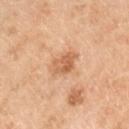{"biopsy_status": "not biopsied; imaged during a skin examination", "site": "right lower leg", "lesion_size": {"long_diameter_mm_approx": 3.5}, "automated_metrics": {"eccentricity": 0.75, "shape_asymmetry": 0.3, "color_variation_0_10": 4.5, "peripheral_color_asymmetry": 1.5, "nevus_likeness_0_100": 0, "lesion_detection_confidence_0_100": 100}, "lighting": "cross-polarized", "image": {"source": "total-body photography crop", "field_of_view_mm": 15}, "patient": {"sex": "male", "age_approx": 65}}